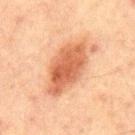workup: imaged on a skin check; not biopsied | subject: male, aged 63 to 67 | image-analysis metrics: a lesion area of about 19 mm² and a shape-asymmetry score of about 0.15 (0 = symmetric); a lesion color around L≈50 a*≈23 b*≈32 in CIELAB, a lesion–skin lightness drop of about 12, and a normalized lesion–skin contrast near 9; a nevus-likeness score of about 95/100 and a lesion-detection confidence of about 100/100 | acquisition: 15 mm crop, total-body photography | lesion diameter: ≈7.5 mm | tile lighting: cross-polarized | anatomic site: the chest.